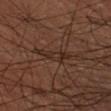Captured during whole-body skin photography for melanoma surveillance; the lesion was not biopsied. A male patient approximately 70 years of age. A lesion tile, about 15 mm wide, cut from a 3D total-body photograph. An algorithmic analysis of the crop reported peripheral color asymmetry of about 0.5. The lesion is on the left forearm. This is a cross-polarized tile.